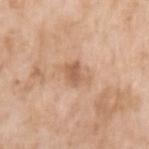| feature | finding |
|---|---|
| biopsy status | total-body-photography surveillance lesion; no biopsy |
| anatomic site | the left upper arm |
| patient | female, in their mid-70s |
| TBP lesion metrics | a footprint of about 4.5 mm², an eccentricity of roughly 0.7, and two-axis asymmetry of about 0.35; a mean CIELAB color near L≈60 a*≈20 b*≈33, roughly 10 lightness units darker than nearby skin, and a lesion-to-skin contrast of about 6.5 (normalized; higher = more distinct); a border-irregularity rating of about 4/10, a color-variation rating of about 2/10, and peripheral color asymmetry of about 0.5; a nevus-likeness score of about 0/100 and a lesion-detection confidence of about 100/100 |
| diameter | about 3 mm |
| illumination | white-light illumination |
| imaging modality | ~15 mm tile from a whole-body skin photo |